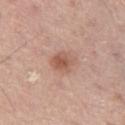biopsy status=imaged on a skin check; not biopsied | patient=male, approximately 55 years of age | image=15 mm crop, total-body photography | site=the left thigh | automated lesion analysis=an average lesion color of about L≈56 a*≈22 b*≈29 (CIELAB), a lesion–skin lightness drop of about 10, and a normalized border contrast of about 7; internal color variation of about 3 on a 0–10 scale and peripheral color asymmetry of about 1; lesion-presence confidence of about 100/100 | illumination=white-light illumination.